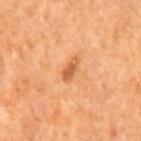Clinical summary: Imaged with cross-polarized lighting. From the mid back. The lesion-visualizer software estimated a lesion area of about 3 mm², a shape eccentricity near 0.9, and a symmetry-axis asymmetry near 0.3. The analysis additionally found a border-irregularity rating of about 3.5/10. The analysis additionally found a nevus-likeness score of about 55/100 and lesion-presence confidence of about 100/100. Measured at roughly 3 mm in maximum diameter. Cropped from a whole-body photographic skin survey; the tile spans about 15 mm. A male patient aged approximately 65.